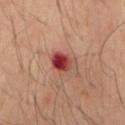biopsy status = catalogued during a skin exam; not biopsied | acquisition = ~15 mm tile from a whole-body skin photo | illumination = cross-polarized | site = the mid back | patient = male, about 50 years old.